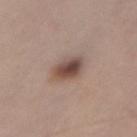<tbp_lesion>
  <biopsy_status>not biopsied; imaged during a skin examination</biopsy_status>
  <site>abdomen</site>
  <lighting>white-light</lighting>
  <image>
    <source>total-body photography crop</source>
    <field_of_view_mm>15</field_of_view_mm>
  </image>
  <patient>
    <sex>female</sex>
    <age_approx>65</age_approx>
  </patient>
  <automated_metrics>
    <shape_asymmetry>0.15</shape_asymmetry>
    <cielab_L>47</cielab_L>
    <cielab_a>17</cielab_a>
    <cielab_b>23</cielab_b>
    <vs_skin_darker_L>14.0</vs_skin_darker_L>
    <nevus_likeness_0_100>95</nevus_likeness_0_100>
    <lesion_detection_confidence_0_100>100</lesion_detection_confidence_0_100>
  </automated_metrics>
</tbp_lesion>